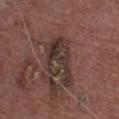Imaged during a routine full-body skin examination; the lesion was not biopsied and no histopathology is available. A region of skin cropped from a whole-body photographic capture, roughly 15 mm wide. The patient is a male in their mid- to late 70s. Measured at roughly 5.5 mm in maximum diameter. The lesion-visualizer software estimated an area of roughly 14 mm², an outline eccentricity of about 0.75 (0 = round, 1 = elongated), and a shape-asymmetry score of about 0.3 (0 = symmetric). And it measured a border-irregularity rating of about 4.5/10, internal color variation of about 8.5 on a 0–10 scale, and a peripheral color-asymmetry measure near 3. Captured under white-light illumination. The lesion is on the left lower leg.